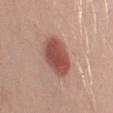This lesion was catalogued during total-body skin photography and was not selected for biopsy. A region of skin cropped from a whole-body photographic capture, roughly 15 mm wide. About 5 mm across. The total-body-photography lesion software estimated a nevus-likeness score of about 100/100 and a lesion-detection confidence of about 100/100. A male patient about 30 years old. From the left upper arm. This is a white-light tile.